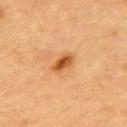Recorded during total-body skin imaging; not selected for excision or biopsy. The subject is a male in their mid-80s or older. The total-body-photography lesion software estimated a lesion area of about 4 mm², a shape eccentricity near 0.8, and a shape-asymmetry score of about 0.25 (0 = symmetric). The analysis additionally found an automated nevus-likeness rating near 95 out of 100 and a detector confidence of about 100 out of 100 that the crop contains a lesion. Measured at roughly 3 mm in maximum diameter. A roughly 15 mm field-of-view crop from a total-body skin photograph. Captured under cross-polarized illumination. The lesion is on the chest.